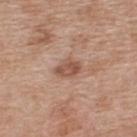Part of a total-body skin-imaging series; this lesion was reviewed on a skin check and was not flagged for biopsy. A 15 mm crop from a total-body photograph taken for skin-cancer surveillance. A female subject in their 40s. The lesion is on the back.